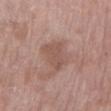Notes:
• workup · total-body-photography surveillance lesion; no biopsy
• body site · the left lower leg
• tile lighting · white-light
• patient · female, roughly 70 years of age
• lesion size · about 4 mm
• imaging modality · ~15 mm crop, total-body skin-cancer survey